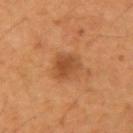The lesion was tiled from a total-body skin photograph and was not biopsied. The lesion's longest dimension is about 4 mm. A 15 mm close-up extracted from a 3D total-body photography capture. A male subject, aged 53–57. Captured under cross-polarized illumination. Automated tile analysis of the lesion measured a lesion color around L≈45 a*≈23 b*≈36 in CIELAB, roughly 9 lightness units darker than nearby skin, and a normalized border contrast of about 7. The analysis additionally found border irregularity of about 2.5 on a 0–10 scale. And it measured a nevus-likeness score of about 75/100 and lesion-presence confidence of about 100/100. Located on the right upper arm.